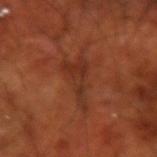Notes:
– biopsy status — total-body-photography surveillance lesion; no biopsy
– image source — 15 mm crop, total-body photography
– subject — male, aged approximately 70
– body site — the arm